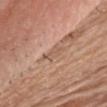Imaged during a routine full-body skin examination; the lesion was not biopsied and no histopathology is available.
From the chest.
The total-body-photography lesion software estimated a nevus-likeness score of about 0/100 and a lesion-detection confidence of about 60/100.
Captured under white-light illumination.
A male patient, aged 78 to 82.
Measured at roughly 2.5 mm in maximum diameter.
A 15 mm crop from a total-body photograph taken for skin-cancer surveillance.